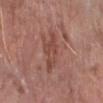lighting: white-light
image:
  source: total-body photography crop
  field_of_view_mm: 15
site: right forearm
patient:
  sex: female
  age_approx: 65